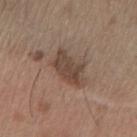Clinical impression:
Recorded during total-body skin imaging; not selected for excision or biopsy.
Acquisition and patient details:
Approximately 5 mm at its widest. A male subject roughly 65 years of age. Cropped from a total-body skin-imaging series; the visible field is about 15 mm. Captured under white-light illumination. The lesion is on the arm.